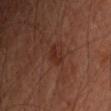<record>
<biopsy_status>not biopsied; imaged during a skin examination</biopsy_status>
<image>
  <source>total-body photography crop</source>
  <field_of_view_mm>15</field_of_view_mm>
</image>
<lesion_size>
  <long_diameter_mm_approx>2.5</long_diameter_mm_approx>
</lesion_size>
<lighting>cross-polarized</lighting>
<patient>
  <sex>male</sex>
  <age_approx>45</age_approx>
</patient>
<site>right upper arm</site>
</record>